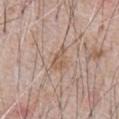The lesion was tiled from a total-body skin photograph and was not biopsied. Approximately 3 mm at its widest. The patient is a male aged around 60. A roughly 15 mm field-of-view crop from a total-body skin photograph. From the abdomen. Captured under white-light illumination.